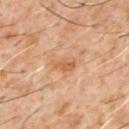Q: Was a biopsy performed?
A: catalogued during a skin exam; not biopsied
Q: What kind of image is this?
A: total-body-photography crop, ~15 mm field of view
Q: What are the patient's age and sex?
A: male, aged around 60
Q: What is the anatomic site?
A: the chest